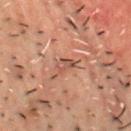No biopsy was performed on this lesion — it was imaged during a full skin examination and was not determined to be concerning. From the front of the torso. This is a cross-polarized tile. This image is a 15 mm lesion crop taken from a total-body photograph. Approximately 2.5 mm at its widest. A male patient in their 50s.